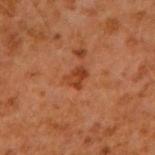notes: catalogued during a skin exam; not biopsied
image source: 15 mm crop, total-body photography
image-analysis metrics: a footprint of about 4 mm², an outline eccentricity of about 0.65 (0 = round, 1 = elongated), and two-axis asymmetry of about 0.2; a mean CIELAB color near L≈40 a*≈27 b*≈36, roughly 8 lightness units darker than nearby skin, and a lesion-to-skin contrast of about 7.5 (normalized; higher = more distinct); a nevus-likeness score of about 20/100 and a lesion-detection confidence of about 100/100
site: the right upper arm
subject: male, about 60 years old
lighting: cross-polarized